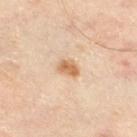<record>
  <biopsy_status>not biopsied; imaged during a skin examination</biopsy_status>
  <image>
    <source>total-body photography crop</source>
    <field_of_view_mm>15</field_of_view_mm>
  </image>
  <site>right thigh</site>
  <patient>
    <age_approx>55</age_approx>
  </patient>
  <lesion_size>
    <long_diameter_mm_approx>2.5</long_diameter_mm_approx>
  </lesion_size>
</record>